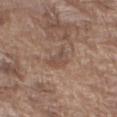Clinical impression: The lesion was photographed on a routine skin check and not biopsied; there is no pathology result. Acquisition and patient details: A region of skin cropped from a whole-body photographic capture, roughly 15 mm wide. The lesion is located on the right upper arm. A male subject aged around 75.